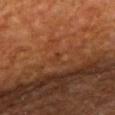| feature | finding |
|---|---|
| biopsy status | catalogued during a skin exam; not biopsied |
| imaging modality | total-body-photography crop, ~15 mm field of view |
| site | the upper back |
| subject | male, about 60 years old |